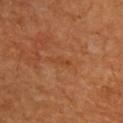Captured under cross-polarized illumination. The recorded lesion diameter is about 2.5 mm. A male patient aged 58 to 62. From the back. Cropped from a total-body skin-imaging series; the visible field is about 15 mm.